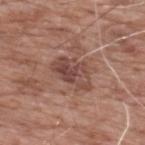Clinical summary: The lesion is on the upper back. The patient is a male about 60 years old. About 5 mm across. An algorithmic analysis of the crop reported a lesion area of about 11 mm² and a shape eccentricity near 0.8. And it measured an automated nevus-likeness rating near 0 out of 100 and a detector confidence of about 100 out of 100 that the crop contains a lesion. Imaged with white-light lighting. Cropped from a total-body skin-imaging series; the visible field is about 15 mm.